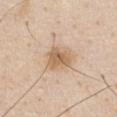The lesion was tiled from a total-body skin photograph and was not biopsied.
The patient is a male aged 58–62.
Cropped from a whole-body photographic skin survey; the tile spans about 15 mm.
The lesion is on the chest.